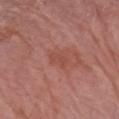This lesion was catalogued during total-body skin photography and was not selected for biopsy. The total-body-photography lesion software estimated an average lesion color of about L≈48 a*≈27 b*≈27 (CIELAB), a lesion–skin lightness drop of about 6, and a normalized lesion–skin contrast near 4.5. The software also gave a border-irregularity rating of about 3.5/10, a color-variation rating of about 1/10, and peripheral color asymmetry of about 0.5. And it measured a lesion-detection confidence of about 100/100. The tile uses white-light illumination. The lesion is located on the left forearm. Approximately 3 mm at its widest. A region of skin cropped from a whole-body photographic capture, roughly 15 mm wide. The patient is a female in their mid-60s.